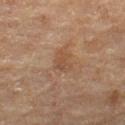The lesion was tiled from a total-body skin photograph and was not biopsied.
Imaged with cross-polarized lighting.
About 2.5 mm across.
A lesion tile, about 15 mm wide, cut from a 3D total-body photograph.
A female patient aged 53 to 57.
From the left thigh.